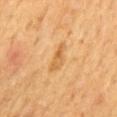biopsy status — total-body-photography surveillance lesion; no biopsy
size — about 3.5 mm
site — the mid back
imaging modality — 15 mm crop, total-body photography
patient — male, about 60 years old